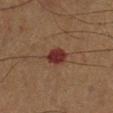The lesion was photographed on a routine skin check and not biopsied; there is no pathology result. Captured under cross-polarized illumination. Automated tile analysis of the lesion measured a footprint of about 5.5 mm², a shape eccentricity near 0.65, and two-axis asymmetry of about 0.25. The analysis additionally found roughly 11 lightness units darker than nearby skin and a lesion-to-skin contrast of about 11.5 (normalized; higher = more distinct). The analysis additionally found border irregularity of about 2.5 on a 0–10 scale, a color-variation rating of about 2/10, and a peripheral color-asymmetry measure near 0.5. The analysis additionally found a nevus-likeness score of about 0/100 and lesion-presence confidence of about 100/100. A male subject aged 53–57. The lesion is on the right lower leg. A region of skin cropped from a whole-body photographic capture, roughly 15 mm wide.